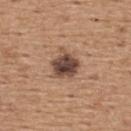biopsy_status: not biopsied; imaged during a skin examination
patient:
  sex: male
  age_approx: 65
lighting: white-light
image:
  source: total-body photography crop
  field_of_view_mm: 15
site: upper back
lesion_size:
  long_diameter_mm_approx: 3.5